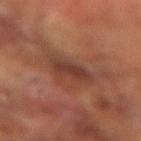  biopsy_status: not biopsied; imaged during a skin examination
  lighting: cross-polarized
  image:
    source: total-body photography crop
    field_of_view_mm: 15
  automated_metrics:
    border_irregularity_0_10: 3.0
    color_variation_0_10: 2.5
    peripheral_color_asymmetry: 1.0
    nevus_likeness_0_100: 25
    lesion_detection_confidence_0_100: 85
  patient:
    sex: male
    age_approx: 65
  site: left forearm